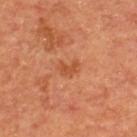Assessment:
Imaged during a routine full-body skin examination; the lesion was not biopsied and no histopathology is available.
Background:
The subject is a male aged 53 to 57. Located on the back. Longest diameter approximately 2.5 mm. The tile uses cross-polarized illumination. A 15 mm close-up extracted from a 3D total-body photography capture.